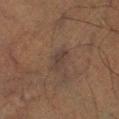Imaged during a routine full-body skin examination; the lesion was not biopsied and no histopathology is available. The total-body-photography lesion software estimated a footprint of about 4.5 mm², a shape eccentricity near 0.85, and two-axis asymmetry of about 0.2. On the right lower leg. Imaged with cross-polarized lighting. A 15 mm close-up tile from a total-body photography series done for melanoma screening. A male subject, aged around 50.